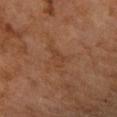<case>
  <biopsy_status>not biopsied; imaged during a skin examination</biopsy_status>
  <site>left forearm</site>
  <lesion_size>
    <long_diameter_mm_approx>3.0</long_diameter_mm_approx>
  </lesion_size>
  <lighting>cross-polarized</lighting>
  <patient>
    <sex>female</sex>
    <age_approx>60</age_approx>
  </patient>
  <image>
    <source>total-body photography crop</source>
    <field_of_view_mm>15</field_of_view_mm>
  </image>
  <automated_metrics>
    <area_mm2_approx>3.5</area_mm2_approx>
    <eccentricity>0.85</eccentricity>
    <shape_asymmetry>0.45</shape_asymmetry>
    <nevus_likeness_0_100>0</nevus_likeness_0_100>
    <lesion_detection_confidence_0_100>100</lesion_detection_confidence_0_100>
  </automated_metrics>
</case>